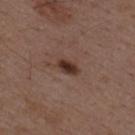| field | value |
|---|---|
| notes | imaged on a skin check; not biopsied |
| lighting | white-light illumination |
| patient | male, roughly 50 years of age |
| lesion size | ≈3 mm |
| anatomic site | the mid back |
| acquisition | total-body-photography crop, ~15 mm field of view |